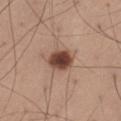notes: total-body-photography surveillance lesion; no biopsy
subject: male, approximately 55 years of age
anatomic site: the left thigh
automated metrics: a mean CIELAB color near L≈44 a*≈20 b*≈27, roughly 17 lightness units darker than nearby skin, and a lesion-to-skin contrast of about 12 (normalized; higher = more distinct); a border-irregularity rating of about 2/10, a color-variation rating of about 4.5/10, and a peripheral color-asymmetry measure near 1
size: ~3.5 mm (longest diameter)
acquisition: total-body-photography crop, ~15 mm field of view